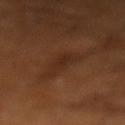Q: Is there a histopathology result?
A: no biopsy performed (imaged during a skin exam)
Q: Where on the body is the lesion?
A: the left lower leg
Q: Patient demographics?
A: male, in their mid- to late 60s
Q: Lesion size?
A: ≈4.5 mm
Q: How was this image acquired?
A: 15 mm crop, total-body photography
Q: What lighting was used for the tile?
A: cross-polarized illumination